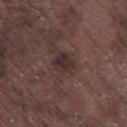biopsy status — total-body-photography surveillance lesion; no biopsy | site — the right lower leg | image — ~15 mm tile from a whole-body skin photo | patient — male, aged approximately 75.